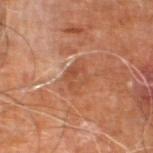The lesion was photographed on a routine skin check and not biopsied; there is no pathology result. A male subject aged around 60. From the leg. Approximately 3.5 mm at its widest. A region of skin cropped from a whole-body photographic capture, roughly 15 mm wide. This is a cross-polarized tile.